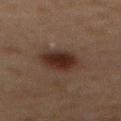No biopsy was performed on this lesion — it was imaged during a full skin examination and was not determined to be concerning. A 15 mm close-up extracted from a 3D total-body photography capture. A male subject aged approximately 75. The lesion is on the abdomen. Approximately 4 mm at its widest. Captured under cross-polarized illumination.